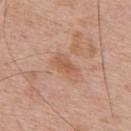Clinical impression: Recorded during total-body skin imaging; not selected for excision or biopsy. Clinical summary: A 15 mm close-up extracted from a 3D total-body photography capture. The patient is a male in their mid- to late 60s. On the upper back.